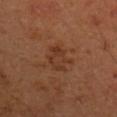Assessment: Imaged during a routine full-body skin examination; the lesion was not biopsied and no histopathology is available. Acquisition and patient details: A 15 mm close-up extracted from a 3D total-body photography capture. Located on the right upper arm. A male subject aged around 45.